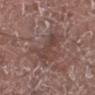Assessment: Recorded during total-body skin imaging; not selected for excision or biopsy. Acquisition and patient details: The patient is a male aged 73–77. Approximately 4 mm at its widest. From the left lower leg. This image is a 15 mm lesion crop taken from a total-body photograph.